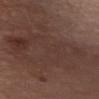Findings:
• notes · imaged on a skin check; not biopsied
• lesion diameter · ~26 mm (longest diameter)
• subject · male, aged around 75
• body site · the chest
• image source · ~15 mm tile from a whole-body skin photo